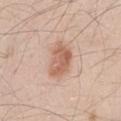Part of a total-body skin-imaging series; this lesion was reviewed on a skin check and was not flagged for biopsy.
A 15 mm close-up extracted from a 3D total-body photography capture.
A male subject, in their mid-40s.
On the right thigh.